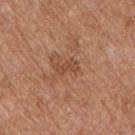notes: imaged on a skin check; not biopsied
subject: male, in their mid-50s
acquisition: total-body-photography crop, ~15 mm field of view
body site: the left upper arm
image-analysis metrics: an eccentricity of roughly 0.85 and a symmetry-axis asymmetry near 0.3; an average lesion color of about L≈48 a*≈23 b*≈31 (CIELAB), a lesion–skin lightness drop of about 8, and a normalized lesion–skin contrast near 6